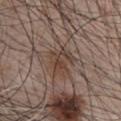• follow-up: catalogued during a skin exam; not biopsied
• diameter: ~3 mm (longest diameter)
• subject: male, about 65 years old
• image: ~15 mm tile from a whole-body skin photo
• automated metrics: roughly 8 lightness units darker than nearby skin
• lighting: white-light
• location: the chest